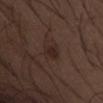Q: Was this lesion biopsied?
A: total-body-photography surveillance lesion; no biopsy
Q: Who is the patient?
A: male, aged approximately 50
Q: Where on the body is the lesion?
A: the abdomen
Q: Lesion size?
A: about 2.5 mm
Q: What lighting was used for the tile?
A: white-light illumination
Q: What is the imaging modality?
A: ~15 mm tile from a whole-body skin photo
Q: What did automated image analysis measure?
A: a classifier nevus-likeness of about 50/100 and a detector confidence of about 100 out of 100 that the crop contains a lesion